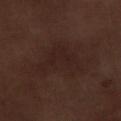<lesion>
  <biopsy_status>not biopsied; imaged during a skin examination</biopsy_status>
  <image>
    <source>total-body photography crop</source>
    <field_of_view_mm>15</field_of_view_mm>
  </image>
  <lesion_size>
    <long_diameter_mm_approx>5.5</long_diameter_mm_approx>
  </lesion_size>
  <patient>
    <sex>male</sex>
    <age_approx>70</age_approx>
  </patient>
  <site>right lower leg</site>
  <automated_metrics>
    <cielab_L>21</cielab_L>
    <cielab_a>16</cielab_a>
    <cielab_b>18</cielab_b>
    <vs_skin_contrast_norm>5.5</vs_skin_contrast_norm>
    <nevus_likeness_0_100>0</nevus_likeness_0_100>
  </automated_metrics>
</lesion>